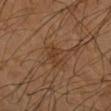The lesion was photographed on a routine skin check and not biopsied; there is no pathology result. Approximately 2.5 mm at its widest. The patient is a male aged 68 to 72. A 15 mm close-up tile from a total-body photography series done for melanoma screening. The lesion is on the left forearm.